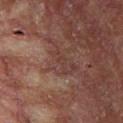Imaged during a routine full-body skin examination; the lesion was not biopsied and no histopathology is available.
The patient is a male roughly 55 years of age.
A 15 mm crop from a total-body photograph taken for skin-cancer surveillance.
On the chest.
The tile uses cross-polarized illumination.